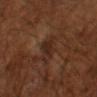Q: Was a biopsy performed?
A: no biopsy performed (imaged during a skin exam)
Q: What did automated image analysis measure?
A: a lesion area of about 5.5 mm² and two-axis asymmetry of about 0.35; a border-irregularity index near 5/10 and radial color variation of about 0.5
Q: Patient demographics?
A: male, aged around 65
Q: Illumination type?
A: cross-polarized illumination
Q: How was this image acquired?
A: ~15 mm tile from a whole-body skin photo
Q: What is the lesion's diameter?
A: ~3.5 mm (longest diameter)